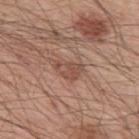Assessment: This lesion was catalogued during total-body skin photography and was not selected for biopsy. Background: From the upper back. A 15 mm close-up extracted from a 3D total-body photography capture. The subject is a male in their mid- to late 50s.